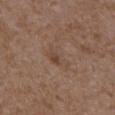Clinical summary: The lesion's longest dimension is about 4.5 mm. The subject is a female aged around 35. A roughly 15 mm field-of-view crop from a total-body skin photograph. Imaged with white-light lighting. The lesion-visualizer software estimated a border-irregularity index near 4/10, internal color variation of about 3.5 on a 0–10 scale, and a peripheral color-asymmetry measure near 1. It also reported a nevus-likeness score of about 0/100 and lesion-presence confidence of about 100/100. The lesion is on the left upper arm.